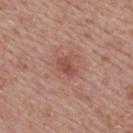Impression:
Imaged during a routine full-body skin examination; the lesion was not biopsied and no histopathology is available.
Clinical summary:
The lesion is located on the back. The recorded lesion diameter is about 2.5 mm. A female patient, aged around 65. This is a white-light tile. The total-body-photography lesion software estimated a lesion color around L≈49 a*≈26 b*≈27 in CIELAB, a lesion–skin lightness drop of about 9, and a lesion-to-skin contrast of about 6.5 (normalized; higher = more distinct). A 15 mm close-up tile from a total-body photography series done for melanoma screening.